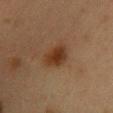{
  "site": "chest",
  "lighting": "cross-polarized",
  "lesion_size": {
    "long_diameter_mm_approx": 3.5
  },
  "automated_metrics": {
    "area_mm2_approx": 8.0,
    "eccentricity": 0.65,
    "shape_asymmetry": 0.25,
    "vs_skin_darker_L": 8.0,
    "vs_skin_contrast_norm": 9.0
  },
  "patient": {
    "sex": "female",
    "age_approx": 40
  },
  "image": {
    "source": "total-body photography crop",
    "field_of_view_mm": 15
  }
}